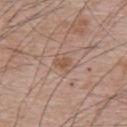This is a white-light tile. A region of skin cropped from a whole-body photographic capture, roughly 15 mm wide. The lesion is on the upper back. The lesion-visualizer software estimated a lesion area of about 3.5 mm², an eccentricity of roughly 0.8, and two-axis asymmetry of about 0.2. The analysis additionally found an average lesion color of about L≈54 a*≈18 b*≈28 (CIELAB). The software also gave lesion-presence confidence of about 100/100. Longest diameter approximately 2.5 mm. A male patient in their mid- to late 60s.